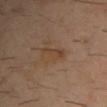• follow-up — total-body-photography surveillance lesion; no biopsy
• subject — male, about 40 years old
• acquisition — ~15 mm crop, total-body skin-cancer survey
• anatomic site — the chest